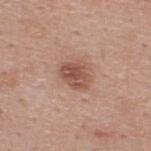Part of a total-body skin-imaging series; this lesion was reviewed on a skin check and was not flagged for biopsy.
A 15 mm crop from a total-body photograph taken for skin-cancer surveillance.
A male patient, aged around 40.
About 3.5 mm across.
On the upper back.